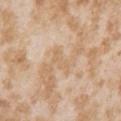The lesion was photographed on a routine skin check and not biopsied; there is no pathology result.
The lesion-visualizer software estimated a lesion area of about 3.5 mm², an outline eccentricity of about 0.9 (0 = round, 1 = elongated), and a shape-asymmetry score of about 0.45 (0 = symmetric). It also reported a mean CIELAB color near L≈66 a*≈16 b*≈35, a lesion–skin lightness drop of about 6, and a normalized lesion–skin contrast near 4.5. The software also gave a border-irregularity rating of about 5/10, a color-variation rating of about 0.5/10, and radial color variation of about 0. And it measured a nevus-likeness score of about 0/100 and lesion-presence confidence of about 100/100.
The patient is a female roughly 25 years of age.
A roughly 15 mm field-of-view crop from a total-body skin photograph.
Imaged with white-light lighting.
The lesion is located on the right upper arm.